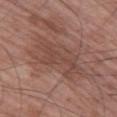Notes:
- workup: total-body-photography surveillance lesion; no biopsy